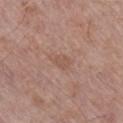| field | value |
|---|---|
| notes | imaged on a skin check; not biopsied |
| image source | ~15 mm tile from a whole-body skin photo |
| lighting | white-light illumination |
| lesion size | about 3 mm |
| site | the right lower leg |
| subject | male, aged around 80 |